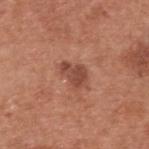{
  "biopsy_status": "not biopsied; imaged during a skin examination",
  "lesion_size": {
    "long_diameter_mm_approx": 3.5
  },
  "image": {
    "source": "total-body photography crop",
    "field_of_view_mm": 15
  },
  "patient": {
    "sex": "male",
    "age_approx": 55
  },
  "lighting": "white-light",
  "site": "upper back"
}